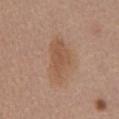Clinical impression:
The lesion was tiled from a total-body skin photograph and was not biopsied.
Context:
The lesion is located on the abdomen. The lesion's longest dimension is about 6 mm. The patient is a male aged around 55. Cropped from a total-body skin-imaging series; the visible field is about 15 mm.